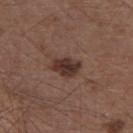Impression: Recorded during total-body skin imaging; not selected for excision or biopsy. Background: A male patient, aged 53 to 57. This is a white-light tile. About 3.5 mm across. The lesion is on the right upper arm. A region of skin cropped from a whole-body photographic capture, roughly 15 mm wide.